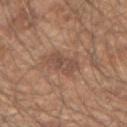biopsy status: imaged on a skin check; not biopsied
subject: male, aged 48 to 52
image: total-body-photography crop, ~15 mm field of view
size: ~3.5 mm (longest diameter)
anatomic site: the right upper arm